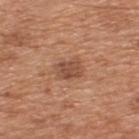The lesion was photographed on a routine skin check and not biopsied; there is no pathology result. A male patient, aged approximately 65. From the upper back. A close-up tile cropped from a whole-body skin photograph, about 15 mm across. The tile uses white-light illumination.